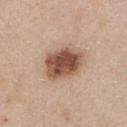This lesion was catalogued during total-body skin photography and was not selected for biopsy. A female patient, aged around 45. Captured under white-light illumination. The lesion's longest dimension is about 5 mm. This image is a 15 mm lesion crop taken from a total-body photograph. Located on the chest.